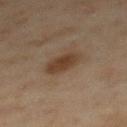| key | value |
|---|---|
| follow-up | total-body-photography surveillance lesion; no biopsy |
| image-analysis metrics | roughly 9 lightness units darker than nearby skin; a within-lesion color-variation index near 2/10 and peripheral color asymmetry of about 0.5; an automated nevus-likeness rating near 95 out of 100 |
| patient | female, about 60 years old |
| acquisition | total-body-photography crop, ~15 mm field of view |
| body site | the back |
| tile lighting | cross-polarized |